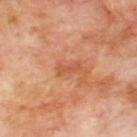Imaged during a routine full-body skin examination; the lesion was not biopsied and no histopathology is available. A lesion tile, about 15 mm wide, cut from a 3D total-body photograph. The total-body-photography lesion software estimated a lesion area of about 2.5 mm², a shape eccentricity near 0.95, and a symmetry-axis asymmetry near 0.3. From the upper back. The patient is a male aged 68–72. Imaged with cross-polarized lighting. Longest diameter approximately 2.5 mm.